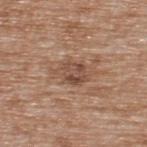Case summary:
* patient: male, about 65 years old
* body site: the upper back
* illumination: white-light illumination
* lesion diameter: about 4 mm
* TBP lesion metrics: an average lesion color of about L≈49 a*≈19 b*≈28 (CIELAB), roughly 9 lightness units darker than nearby skin, and a lesion-to-skin contrast of about 7 (normalized; higher = more distinct); a nevus-likeness score of about 0/100 and a lesion-detection confidence of about 95/100
* image: total-body-photography crop, ~15 mm field of view